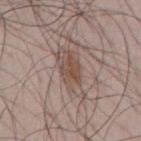Q: Was a biopsy performed?
A: catalogued during a skin exam; not biopsied
Q: What is the imaging modality?
A: total-body-photography crop, ~15 mm field of view
Q: Where on the body is the lesion?
A: the chest
Q: How was the tile lit?
A: white-light
Q: Who is the patient?
A: male, aged approximately 45